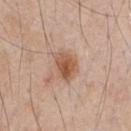Notes:
• location: the chest
• patient: male, aged around 65
• image: 15 mm crop, total-body photography